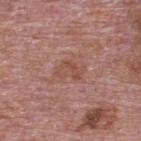Q: Was a biopsy performed?
A: catalogued during a skin exam; not biopsied
Q: How large is the lesion?
A: about 3.5 mm
Q: Lesion location?
A: the back
Q: What kind of image is this?
A: ~15 mm tile from a whole-body skin photo
Q: Patient demographics?
A: male, in their mid-70s